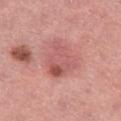notes: imaged on a skin check; not biopsied
image source: ~15 mm crop, total-body skin-cancer survey
location: the left thigh
lesion diameter: about 4.5 mm
subject: female, aged approximately 45
tile lighting: white-light illumination